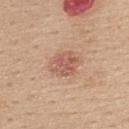• biopsy status · imaged on a skin check; not biopsied
• location · the upper back
• patient · female, approximately 45 years of age
• tile lighting · white-light
• image source · total-body-photography crop, ~15 mm field of view